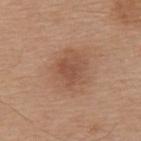Q: Is there a histopathology result?
A: total-body-photography surveillance lesion; no biopsy
Q: What did automated image analysis measure?
A: a normalized lesion–skin contrast near 5.5; a within-lesion color-variation index near 2.5/10 and radial color variation of about 1; an automated nevus-likeness rating near 25 out of 100
Q: What are the patient's age and sex?
A: male, aged 63 to 67
Q: Illumination type?
A: white-light
Q: What is the lesion's diameter?
A: about 3.5 mm
Q: What is the anatomic site?
A: the upper back
Q: What kind of image is this?
A: ~15 mm crop, total-body skin-cancer survey